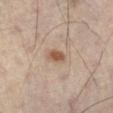Acquisition and patient details:
The total-body-photography lesion software estimated a within-lesion color-variation index near 2.5/10 and a peripheral color-asymmetry measure near 1. The analysis additionally found a classifier nevus-likeness of about 90/100 and a lesion-detection confidence of about 100/100. This is a cross-polarized tile. On the left leg. A 15 mm close-up extracted from a 3D total-body photography capture. The patient is a male aged around 50. Approximately 2.5 mm at its widest.